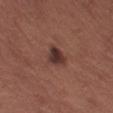| field | value |
|---|---|
| biopsy status | total-body-photography surveillance lesion; no biopsy |
| imaging modality | ~15 mm crop, total-body skin-cancer survey |
| TBP lesion metrics | a footprint of about 5 mm²; a mean CIELAB color near L≈34 a*≈20 b*≈21, a lesion–skin lightness drop of about 11, and a lesion-to-skin contrast of about 10.5 (normalized; higher = more distinct) |
| body site | the left thigh |
| patient | female, aged around 65 |
| tile lighting | white-light |